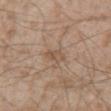Assessment:
No biopsy was performed on this lesion — it was imaged during a full skin examination and was not determined to be concerning.
Background:
This is a white-light tile. From the front of the torso. An algorithmic analysis of the crop reported an area of roughly 3.5 mm², an eccentricity of roughly 0.85, and a symmetry-axis asymmetry near 0.2. And it measured a classifier nevus-likeness of about 0/100 and a lesion-detection confidence of about 100/100. The recorded lesion diameter is about 2.5 mm. A lesion tile, about 15 mm wide, cut from a 3D total-body photograph. A male patient roughly 55 years of age.